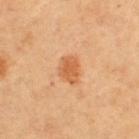biopsy status: no biopsy performed (imaged during a skin exam)
location: the left upper arm
automated lesion analysis: an area of roughly 5.5 mm², a shape eccentricity near 0.6, and a symmetry-axis asymmetry near 0.25; border irregularity of about 2.5 on a 0–10 scale and a peripheral color-asymmetry measure near 1; a nevus-likeness score of about 95/100
image: total-body-photography crop, ~15 mm field of view
lighting: cross-polarized
patient: female, aged around 65